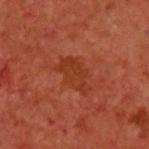Captured during whole-body skin photography for melanoma surveillance; the lesion was not biopsied. A lesion tile, about 15 mm wide, cut from a 3D total-body photograph. On the upper back. Approximately 4.5 mm at its widest. The total-body-photography lesion software estimated a mean CIELAB color near L≈35 a*≈30 b*≈35, roughly 6 lightness units darker than nearby skin, and a normalized lesion–skin contrast near 6. The analysis additionally found a border-irregularity rating of about 4/10, internal color variation of about 2.5 on a 0–10 scale, and radial color variation of about 1. A male subject, in their 60s. The tile uses cross-polarized illumination.